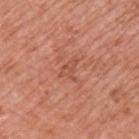Part of a total-body skin-imaging series; this lesion was reviewed on a skin check and was not flagged for biopsy. A close-up tile cropped from a whole-body skin photograph, about 15 mm across. A male subject aged 68–72. Captured under white-light illumination. The recorded lesion diameter is about 3 mm. Automated image analysis of the tile measured a lesion area of about 3.5 mm², a shape eccentricity near 0.7, and a shape-asymmetry score of about 0.45 (0 = symmetric). And it measured an average lesion color of about L≈53 a*≈27 b*≈32 (CIELAB) and a lesion-to-skin contrast of about 4.5 (normalized; higher = more distinct). And it measured a border-irregularity index near 5.5/10, a color-variation rating of about 1.5/10, and peripheral color asymmetry of about 0.5. From the arm.